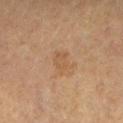A 15 mm close-up extracted from a 3D total-body photography capture. Located on the right forearm. The tile uses cross-polarized illumination. A female subject aged approximately 65.